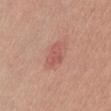Part of a total-body skin-imaging series; this lesion was reviewed on a skin check and was not flagged for biopsy.